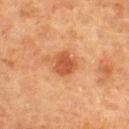Impression:
Imaged during a routine full-body skin examination; the lesion was not biopsied and no histopathology is available.
Background:
The patient is a female aged approximately 55. This image is a 15 mm lesion crop taken from a total-body photograph. Located on the upper back. The tile uses cross-polarized illumination. Measured at roughly 3 mm in maximum diameter.